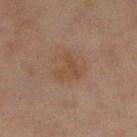Recorded during total-body skin imaging; not selected for excision or biopsy. This is a cross-polarized tile. A female patient aged 68–72. The lesion is located on the right lower leg. Approximately 3 mm at its widest. Cropped from a whole-body photographic skin survey; the tile spans about 15 mm. The lesion-visualizer software estimated a classifier nevus-likeness of about 0/100.